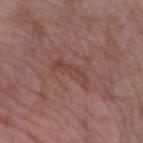Recorded during total-body skin imaging; not selected for excision or biopsy. A male subject in their 40s. The total-body-photography lesion software estimated a classifier nevus-likeness of about 0/100 and lesion-presence confidence of about 100/100. A lesion tile, about 15 mm wide, cut from a 3D total-body photograph. The lesion is on the left forearm. This is a white-light tile. The recorded lesion diameter is about 4.5 mm.